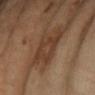Clinical impression: The lesion was tiled from a total-body skin photograph and was not biopsied.